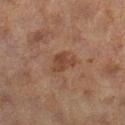Clinical impression: The lesion was photographed on a routine skin check and not biopsied; there is no pathology result. Acquisition and patient details: Located on the right lower leg. A region of skin cropped from a whole-body photographic capture, roughly 15 mm wide. Captured under cross-polarized illumination. A female patient, aged approximately 60.